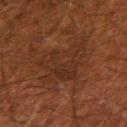<case>
  <biopsy_status>not biopsied; imaged during a skin examination</biopsy_status>
  <patient>
    <sex>male</sex>
    <age_approx>50</age_approx>
  </patient>
  <image>
    <source>total-body photography crop</source>
    <field_of_view_mm>15</field_of_view_mm>
  </image>
  <site>right upper arm</site>
</case>